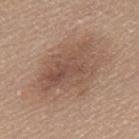Notes:
• anatomic site — the mid back
• TBP lesion metrics — a within-lesion color-variation index near 5/10 and peripheral color asymmetry of about 1.5; an automated nevus-likeness rating near 35 out of 100 and lesion-presence confidence of about 100/100
• image — total-body-photography crop, ~15 mm field of view
• illumination — white-light illumination
• size — ≈8 mm
• patient — female, aged around 65